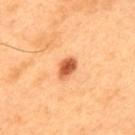Assessment: The lesion was photographed on a routine skin check and not biopsied; there is no pathology result. Background: Located on the upper back. A region of skin cropped from a whole-body photographic capture, roughly 15 mm wide. A male subject, aged approximately 50. Approximately 3 mm at its widest. Captured under cross-polarized illumination. The lesion-visualizer software estimated a border-irregularity index near 1.5/10, a color-variation rating of about 4/10, and peripheral color asymmetry of about 1. The analysis additionally found an automated nevus-likeness rating near 100 out of 100.